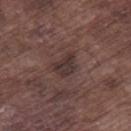Clinical impression: The lesion was photographed on a routine skin check and not biopsied; there is no pathology result. Image and clinical context: Captured under white-light illumination. The lesion is on the leg. A male subject aged 73–77. This image is a 15 mm lesion crop taken from a total-body photograph.